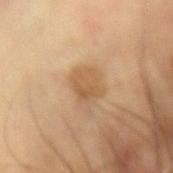biopsy status — catalogued during a skin exam; not biopsied
site — the left arm
patient — male, aged around 60
acquisition — 15 mm crop, total-body photography
diameter — ≈3 mm
tile lighting — cross-polarized